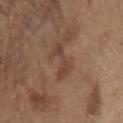Part of a total-body skin-imaging series; this lesion was reviewed on a skin check and was not flagged for biopsy. The subject is a male approximately 70 years of age. Located on the left forearm. A 15 mm crop from a total-body photograph taken for skin-cancer surveillance.